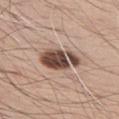Clinical impression:
The lesion was photographed on a routine skin check and not biopsied; there is no pathology result.
Acquisition and patient details:
A male patient, in their 70s. Cropped from a total-body skin-imaging series; the visible field is about 15 mm. The lesion is on the upper back. An algorithmic analysis of the crop reported a mean CIELAB color near L≈48 a*≈17 b*≈24, about 19 CIELAB-L* units darker than the surrounding skin, and a normalized border contrast of about 12.5. The analysis additionally found an automated nevus-likeness rating near 90 out of 100 and a detector confidence of about 100 out of 100 that the crop contains a lesion. This is a white-light tile.